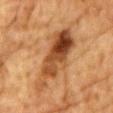| field | value |
|---|---|
| body site | the chest |
| size | about 9.5 mm |
| patient | male, aged approximately 85 or older |
| image | 15 mm crop, total-body photography |